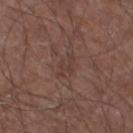The lesion was photographed on a routine skin check and not biopsied; there is no pathology result. The tile uses white-light illumination. Approximately 3.5 mm at its widest. The total-body-photography lesion software estimated a border-irregularity index near 8/10, a color-variation rating of about 0/10, and peripheral color asymmetry of about 0. It also reported a classifier nevus-likeness of about 0/100 and a lesion-detection confidence of about 75/100. A male patient, in their mid-60s. The lesion is on the left lower leg. A region of skin cropped from a whole-body photographic capture, roughly 15 mm wide.